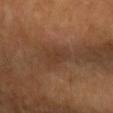The lesion was photographed on a routine skin check and not biopsied; there is no pathology result. A female subject aged approximately 70. Located on the right forearm. Automated tile analysis of the lesion measured a mean CIELAB color near L≈38 a*≈19 b*≈30, about 5 CIELAB-L* units darker than the surrounding skin, and a lesion-to-skin contrast of about 4.5 (normalized; higher = more distinct). It also reported a border-irregularity rating of about 3/10, a color-variation rating of about 1.5/10, and radial color variation of about 0.5. A roughly 15 mm field-of-view crop from a total-body skin photograph. About 3.5 mm across.